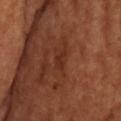Q: Was a biopsy performed?
A: imaged on a skin check; not biopsied
Q: What did automated image analysis measure?
A: border irregularity of about 4.5 on a 0–10 scale, internal color variation of about 0 on a 0–10 scale, and a peripheral color-asymmetry measure near 0
Q: Where on the body is the lesion?
A: the head or neck
Q: Illumination type?
A: cross-polarized
Q: How was this image acquired?
A: ~15 mm crop, total-body skin-cancer survey